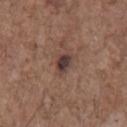The lesion was photographed on a routine skin check and not biopsied; there is no pathology result. The subject is a male aged 68 to 72. Automated tile analysis of the lesion measured an area of roughly 5 mm² and an eccentricity of roughly 0.7. And it measured a normalized border contrast of about 10. It also reported a border-irregularity rating of about 2.5/10 and radial color variation of about 1.5. It also reported a classifier nevus-likeness of about 25/100 and a detector confidence of about 100 out of 100 that the crop contains a lesion. Imaged with white-light lighting. The lesion is located on the chest. A roughly 15 mm field-of-view crop from a total-body skin photograph.